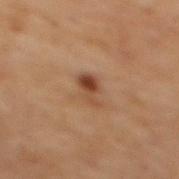No biopsy was performed on this lesion — it was imaged during a full skin examination and was not determined to be concerning.
On the mid back.
The patient is a male aged approximately 70.
Automated image analysis of the tile measured a lesion color around L≈34 a*≈17 b*≈26 in CIELAB, a lesion–skin lightness drop of about 8, and a normalized border contrast of about 8. It also reported a border-irregularity rating of about 4/10 and peripheral color asymmetry of about 2. It also reported a nevus-likeness score of about 90/100 and lesion-presence confidence of about 100/100.
Measured at roughly 3.5 mm in maximum diameter.
A close-up tile cropped from a whole-body skin photograph, about 15 mm across.
This is a cross-polarized tile.